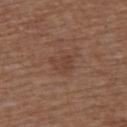<case>
<lighting>white-light</lighting>
<image>
  <source>total-body photography crop</source>
  <field_of_view_mm>15</field_of_view_mm>
</image>
<site>back</site>
<patient>
  <sex>female</sex>
  <age_approx>75</age_approx>
</patient>
<lesion_size>
  <long_diameter_mm_approx>3.0</long_diameter_mm_approx>
</lesion_size>
</case>